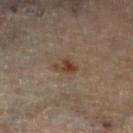Background: The lesion is located on the left lower leg. Automated image analysis of the tile measured a border-irregularity rating of about 4/10, a within-lesion color-variation index near 2.5/10, and radial color variation of about 1. The software also gave a classifier nevus-likeness of about 55/100 and lesion-presence confidence of about 100/100. About 3 mm across. A 15 mm crop from a total-body photograph taken for skin-cancer surveillance. A female subject, in their 60s. Captured under cross-polarized illumination.